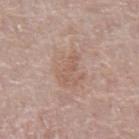The lesion was photographed on a routine skin check and not biopsied; there is no pathology result.
A 15 mm close-up tile from a total-body photography series done for melanoma screening.
The patient is a male about 70 years old.
Captured under white-light illumination.
From the abdomen.